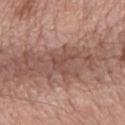Impression:
The lesion was tiled from a total-body skin photograph and was not biopsied.
Background:
The subject is a male approximately 75 years of age. Located on the back. A close-up tile cropped from a whole-body skin photograph, about 15 mm across.